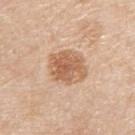Part of a total-body skin-imaging series; this lesion was reviewed on a skin check and was not flagged for biopsy.
The patient is a male aged 78–82.
About 4.5 mm across.
Imaged with white-light lighting.
A close-up tile cropped from a whole-body skin photograph, about 15 mm across.
The lesion is located on the right upper arm.
Automated image analysis of the tile measured an area of roughly 14 mm² and a symmetry-axis asymmetry near 0.15. The software also gave a border-irregularity rating of about 1.5/10, a color-variation rating of about 4.5/10, and radial color variation of about 1.5.